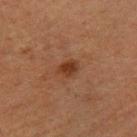Image and clinical context:
The lesion is located on the right forearm. Imaged with cross-polarized lighting. A lesion tile, about 15 mm wide, cut from a 3D total-body photograph. Longest diameter approximately 2.5 mm. The patient is a female aged approximately 40.